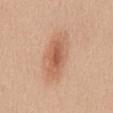tile lighting — white-light illumination
imaging modality — ~15 mm crop, total-body skin-cancer survey
size — ~6.5 mm (longest diameter)
anatomic site — the mid back
patient — female, aged 43 to 47